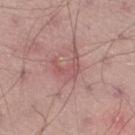{"biopsy_status": "not biopsied; imaged during a skin examination"}